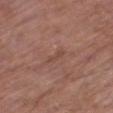Assessment:
No biopsy was performed on this lesion — it was imaged during a full skin examination and was not determined to be concerning.
Clinical summary:
A 15 mm close-up extracted from a 3D total-body photography capture. The total-body-photography lesion software estimated a mean CIELAB color near L≈46 a*≈21 b*≈26 and a normalized lesion–skin contrast near 4.5. The software also gave an automated nevus-likeness rating near 0 out of 100 and lesion-presence confidence of about 90/100. On the leg. A male patient aged approximately 65. The tile uses white-light illumination.